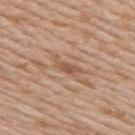The lesion is located on the upper back.
This is a white-light tile.
A female subject, in their mid-40s.
A roughly 15 mm field-of-view crop from a total-body skin photograph.
The lesion's longest dimension is about 3.5 mm.